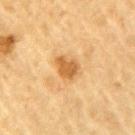{
  "biopsy_status": "not biopsied; imaged during a skin examination",
  "image": {
    "source": "total-body photography crop",
    "field_of_view_mm": 15
  },
  "patient": {
    "sex": "male",
    "age_approx": "85+"
  },
  "lesion_size": {
    "long_diameter_mm_approx": 3.0
  },
  "lighting": "cross-polarized",
  "site": "left upper arm"
}